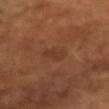| key | value |
|---|---|
| notes | no biopsy performed (imaged during a skin exam) |
| automated lesion analysis | a border-irregularity rating of about 4/10, a color-variation rating of about 1/10, and a peripheral color-asymmetry measure near 0.5 |
| patient | male, aged around 65 |
| image source | 15 mm crop, total-body photography |